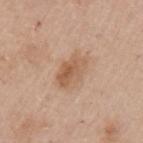notes: imaged on a skin check; not biopsied
tile lighting: white-light
lesion diameter: ~4 mm (longest diameter)
location: the left upper arm
patient: female, aged 58 to 62
image source: total-body-photography crop, ~15 mm field of view